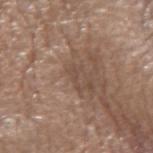No biopsy was performed on this lesion — it was imaged during a full skin examination and was not determined to be concerning.
The lesion is on the arm.
The patient is a female aged 73 to 77.
The recorded lesion diameter is about 3 mm.
Automated tile analysis of the lesion measured a lesion area of about 2 mm² and a symmetry-axis asymmetry near 0.7. The analysis additionally found a lesion color around L≈47 a*≈17 b*≈25 in CIELAB and about 6 CIELAB-L* units darker than the surrounding skin. And it measured lesion-presence confidence of about 50/100.
This is a white-light tile.
Cropped from a whole-body photographic skin survey; the tile spans about 15 mm.